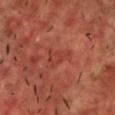The lesion was photographed on a routine skin check and not biopsied; there is no pathology result.
The tile uses cross-polarized illumination.
Automated tile analysis of the lesion measured an automated nevus-likeness rating near 0 out of 100 and a lesion-detection confidence of about 100/100.
A male subject aged 53 to 57.
The lesion is on the front of the torso.
A 15 mm crop from a total-body photograph taken for skin-cancer surveillance.
About 3.5 mm across.